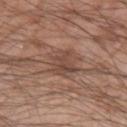Assessment:
Captured during whole-body skin photography for melanoma surveillance; the lesion was not biopsied.
Acquisition and patient details:
This is a white-light tile. The patient is a male roughly 75 years of age. A region of skin cropped from a whole-body photographic capture, roughly 15 mm wide. The lesion is on the left upper arm.